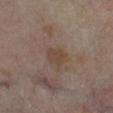The lesion was photographed on a routine skin check and not biopsied; there is no pathology result.
The lesion-visualizer software estimated a lesion area of about 4.5 mm² and an outline eccentricity of about 0.6 (0 = round, 1 = elongated). The analysis additionally found a mean CIELAB color near L≈41 a*≈14 b*≈24, roughly 6 lightness units darker than nearby skin, and a normalized lesion–skin contrast near 6. The analysis additionally found border irregularity of about 2.5 on a 0–10 scale and a color-variation rating of about 1.5/10.
A male patient, aged 68 to 72.
About 2.5 mm across.
From the right lower leg.
A roughly 15 mm field-of-view crop from a total-body skin photograph.
Imaged with cross-polarized lighting.